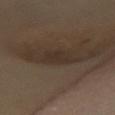Recorded during total-body skin imaging; not selected for excision or biopsy.
The subject is a female approximately 60 years of age.
A 15 mm close-up tile from a total-body photography series done for melanoma screening.
The recorded lesion diameter is about 3 mm.
On the front of the torso.